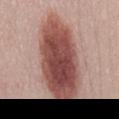No biopsy was performed on this lesion — it was imaged during a full skin examination and was not determined to be concerning. The recorded lesion diameter is about 11 mm. The subject is a female aged 48–52. Imaged with white-light lighting. The lesion is on the mid back. The total-body-photography lesion software estimated a footprint of about 48 mm². The analysis additionally found a border-irregularity index near 2/10, a color-variation rating of about 7/10, and a peripheral color-asymmetry measure near 2.5. It also reported a lesion-detection confidence of about 100/100. Cropped from a total-body skin-imaging series; the visible field is about 15 mm.